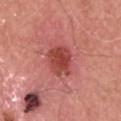Q: Automated lesion metrics?
A: a symmetry-axis asymmetry near 0.2; a mean CIELAB color near L≈46 a*≈34 b*≈28, a lesion–skin lightness drop of about 12, and a normalized border contrast of about 8.5
Q: How was this image acquired?
A: ~15 mm crop, total-body skin-cancer survey
Q: Lesion size?
A: ≈3.5 mm
Q: What lighting was used for the tile?
A: white-light
Q: Where on the body is the lesion?
A: the head or neck
Q: Patient demographics?
A: male, aged approximately 60